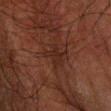Findings:
- workup · catalogued during a skin exam; not biopsied